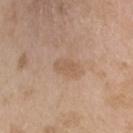Clinical impression: Imaged during a routine full-body skin examination; the lesion was not biopsied and no histopathology is available. Image and clinical context: A 15 mm close-up tile from a total-body photography series done for melanoma screening. From the head or neck. A male subject aged 23 to 27. Captured under white-light illumination. Automated image analysis of the tile measured a mean CIELAB color near L≈57 a*≈17 b*≈31 and a normalized lesion–skin contrast near 5. The software also gave border irregularity of about 2 on a 0–10 scale, internal color variation of about 1.5 on a 0–10 scale, and radial color variation of about 0.5. It also reported a detector confidence of about 100 out of 100 that the crop contains a lesion.